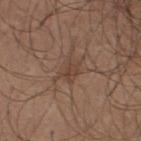Findings:
– follow-up · catalogued during a skin exam; not biopsied
– size · about 2.5 mm
– site · the upper back
– subject · male, aged 23 to 27
– image source · ~15 mm tile from a whole-body skin photo